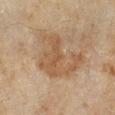notes = no biopsy performed (imaged during a skin exam); anatomic site = the left lower leg; lighting = cross-polarized; lesion size = ~7 mm (longest diameter); acquisition = ~15 mm crop, total-body skin-cancer survey; subject = female, roughly 60 years of age.